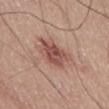Captured during whole-body skin photography for melanoma surveillance; the lesion was not biopsied.
The patient is a male aged 53 to 57.
Approximately 4 mm at its widest.
The tile uses white-light illumination.
From the abdomen.
A 15 mm crop from a total-body photograph taken for skin-cancer surveillance.